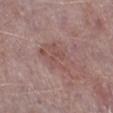Impression:
The lesion was photographed on a routine skin check and not biopsied; there is no pathology result.
Acquisition and patient details:
Measured at roughly 5 mm in maximum diameter. On the leg. A male subject approximately 75 years of age. The total-body-photography lesion software estimated a mean CIELAB color near L≈51 a*≈19 b*≈22 and about 6 CIELAB-L* units darker than the surrounding skin. The software also gave a border-irregularity rating of about 3/10, a within-lesion color-variation index near 3/10, and a peripheral color-asymmetry measure near 1. The software also gave a classifier nevus-likeness of about 0/100 and a lesion-detection confidence of about 100/100. Imaged with white-light lighting. A close-up tile cropped from a whole-body skin photograph, about 15 mm across.